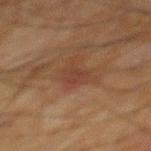Impression: No biopsy was performed on this lesion — it was imaged during a full skin examination and was not determined to be concerning. Background: The patient is a male aged 63 to 67. A roughly 15 mm field-of-view crop from a total-body skin photograph. On the upper back. Imaged with cross-polarized lighting. Approximately 2.5 mm at its widest.